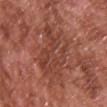| key | value |
|---|---|
| notes | no biopsy performed (imaged during a skin exam) |
| automated metrics | a mean CIELAB color near L≈43 a*≈26 b*≈28, a lesion–skin lightness drop of about 7, and a normalized border contrast of about 5; border irregularity of about 4.5 on a 0–10 scale, a within-lesion color-variation index near 4.5/10, and peripheral color asymmetry of about 1.5 |
| lesion diameter | ~6.5 mm (longest diameter) |
| subject | male, approximately 65 years of age |
| anatomic site | the chest |
| illumination | white-light |
| acquisition | ~15 mm tile from a whole-body skin photo |